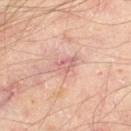Q: Was this lesion biopsied?
A: total-body-photography surveillance lesion; no biopsy
Q: How was the tile lit?
A: cross-polarized illumination
Q: What is the anatomic site?
A: the left thigh
Q: What is the imaging modality?
A: ~15 mm tile from a whole-body skin photo
Q: Patient demographics?
A: aged approximately 55
Q: What is the lesion's diameter?
A: ≈3 mm